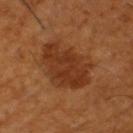Findings:
* subject: male, roughly 60 years of age
* site: the left thigh
* illumination: cross-polarized illumination
* acquisition: ~15 mm tile from a whole-body skin photo